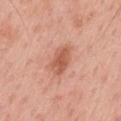Q: What are the patient's age and sex?
A: male, aged 58–62
Q: Illumination type?
A: white-light
Q: What is the lesion's diameter?
A: about 4 mm
Q: How was this image acquired?
A: total-body-photography crop, ~15 mm field of view
Q: Where on the body is the lesion?
A: the mid back